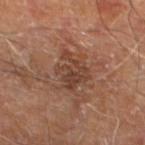Recorded during total-body skin imaging; not selected for excision or biopsy. On the left lower leg. The lesion-visualizer software estimated a shape eccentricity near 0.6 and a shape-asymmetry score of about 0.3 (0 = symmetric). Approximately 5 mm at its widest. The tile uses cross-polarized illumination. A region of skin cropped from a whole-body photographic capture, roughly 15 mm wide. A male patient aged 68–72.